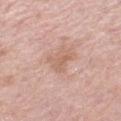Assessment: This lesion was catalogued during total-body skin photography and was not selected for biopsy. Clinical summary: A female subject, about 70 years old. From the left thigh. The recorded lesion diameter is about 3.5 mm. Cropped from a whole-body photographic skin survey; the tile spans about 15 mm.